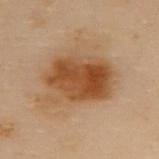Clinical summary:
A female subject aged approximately 60. Cropped from a total-body skin-imaging series; the visible field is about 15 mm. The lesion is located on the upper back. Longest diameter approximately 7 mm. Imaged with cross-polarized lighting.